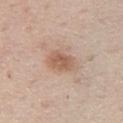Q: Was this lesion biopsied?
A: catalogued during a skin exam; not biopsied
Q: What are the patient's age and sex?
A: male, in their 60s
Q: Lesion location?
A: the chest
Q: How large is the lesion?
A: ≈3.5 mm
Q: Automated lesion metrics?
A: a within-lesion color-variation index near 3/10 and radial color variation of about 1; a nevus-likeness score of about 80/100
Q: How was the tile lit?
A: white-light
Q: How was this image acquired?
A: ~15 mm crop, total-body skin-cancer survey